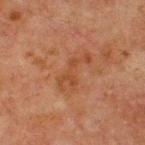Q: What is the lesion's diameter?
A: about 5 mm
Q: Automated lesion metrics?
A: a lesion area of about 7 mm², an outline eccentricity of about 0.9 (0 = round, 1 = elongated), and a shape-asymmetry score of about 0.6 (0 = symmetric); a classifier nevus-likeness of about 0/100
Q: Where on the body is the lesion?
A: the chest
Q: What is the imaging modality?
A: ~15 mm tile from a whole-body skin photo
Q: What are the patient's age and sex?
A: male, about 65 years old